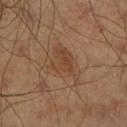Clinical impression:
The lesion was photographed on a routine skin check and not biopsied; there is no pathology result.
Acquisition and patient details:
A roughly 15 mm field-of-view crop from a total-body skin photograph. The recorded lesion diameter is about 5 mm. The patient is a male aged around 45. From the right lower leg.